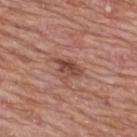Cropped from a whole-body photographic skin survey; the tile spans about 15 mm. The lesion's longest dimension is about 4 mm. The subject is a female in their 60s. From the upper back.